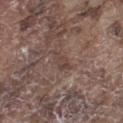Part of a total-body skin-imaging series; this lesion was reviewed on a skin check and was not flagged for biopsy. A male patient, roughly 75 years of age. A roughly 15 mm field-of-view crop from a total-body skin photograph. On the left thigh.